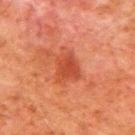No biopsy was performed on this lesion — it was imaged during a full skin examination and was not determined to be concerning.
Cropped from a total-body skin-imaging series; the visible field is about 15 mm.
The subject is a male aged 78 to 82.
Measured at roughly 3.5 mm in maximum diameter.
Located on the upper back.
The tile uses cross-polarized illumination.